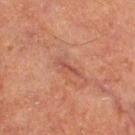| feature | finding |
|---|---|
| biopsy status | catalogued during a skin exam; not biopsied |
| subject | male, aged 63 to 67 |
| image-analysis metrics | a lesion area of about 3.5 mm² and a symmetry-axis asymmetry near 0.3; a mean CIELAB color near L≈41 a*≈22 b*≈24 and about 6 CIELAB-L* units darker than the surrounding skin; peripheral color asymmetry of about 0; an automated nevus-likeness rating near 0 out of 100 and a detector confidence of about 90 out of 100 that the crop contains a lesion |
| size | ~3.5 mm (longest diameter) |
| body site | the left thigh |
| tile lighting | cross-polarized |
| imaging modality | ~15 mm tile from a whole-body skin photo |